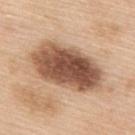A female subject aged around 55.
A roughly 15 mm field-of-view crop from a total-body skin photograph.
The lesion is on the back.